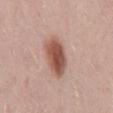follow-up: catalogued during a skin exam; not biopsied
location: the mid back
acquisition: 15 mm crop, total-body photography
patient: male, aged 38 to 42
diameter: about 5.5 mm
automated metrics: a lesion area of about 13 mm², a shape eccentricity near 0.85, and two-axis asymmetry of about 0.15; a lesion-to-skin contrast of about 10 (normalized; higher = more distinct); a border-irregularity index near 1.5/10; an automated nevus-likeness rating near 100 out of 100 and a detector confidence of about 100 out of 100 that the crop contains a lesion